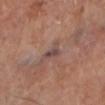{"biopsy_status": "not biopsied; imaged during a skin examination", "site": "left lower leg", "automated_metrics": {"area_mm2_approx": 3.5, "shape_asymmetry": 0.45, "cielab_L": 46, "cielab_a": 19, "cielab_b": 21, "vs_skin_darker_L": 8.0, "nevus_likeness_0_100": 0}, "lesion_size": {"long_diameter_mm_approx": 2.5}, "image": {"source": "total-body photography crop", "field_of_view_mm": 15}, "lighting": "cross-polarized"}